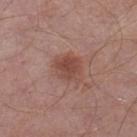Captured during whole-body skin photography for melanoma surveillance; the lesion was not biopsied. Cropped from a total-body skin-imaging series; the visible field is about 15 mm. A male patient, about 55 years old. Longest diameter approximately 3.5 mm. The lesion-visualizer software estimated a footprint of about 8 mm², an eccentricity of roughly 0.55, and a symmetry-axis asymmetry near 0.2. It also reported a lesion color around L≈47 a*≈22 b*≈25 in CIELAB and a lesion–skin lightness drop of about 9. On the right lower leg.